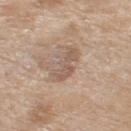No biopsy was performed on this lesion — it was imaged during a full skin examination and was not determined to be concerning.
Captured under white-light illumination.
The recorded lesion diameter is about 4.5 mm.
The lesion-visualizer software estimated a footprint of about 7.5 mm², an eccentricity of roughly 0.9, and a symmetry-axis asymmetry near 0.35. And it measured a lesion-to-skin contrast of about 6 (normalized; higher = more distinct).
The lesion is on the right thigh.
A roughly 15 mm field-of-view crop from a total-body skin photograph.
A female subject approximately 75 years of age.